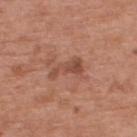No biopsy was performed on this lesion — it was imaged during a full skin examination and was not determined to be concerning. A male subject aged 68 to 72. Located on the upper back. A close-up tile cropped from a whole-body skin photograph, about 15 mm across.